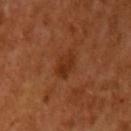notes: imaged on a skin check; not biopsied
location: the left upper arm
patient: female, approximately 55 years of age
acquisition: ~15 mm tile from a whole-body skin photo
TBP lesion metrics: an average lesion color of about L≈33 a*≈26 b*≈35 (CIELAB); an automated nevus-likeness rating near 25 out of 100